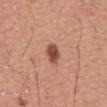The lesion was photographed on a routine skin check and not biopsied; there is no pathology result. The lesion is on the back. Automated image analysis of the tile measured a shape eccentricity near 0.6 and a symmetry-axis asymmetry near 0.15. The analysis additionally found a border-irregularity index near 1.5/10, a color-variation rating of about 3.5/10, and a peripheral color-asymmetry measure near 1. A male subject, roughly 45 years of age. Cropped from a whole-body photographic skin survey; the tile spans about 15 mm.